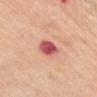Q: Was a biopsy performed?
A: imaged on a skin check; not biopsied
Q: What kind of image is this?
A: ~15 mm crop, total-body skin-cancer survey
Q: Who is the patient?
A: female, aged 63–67
Q: Where on the body is the lesion?
A: the front of the torso
Q: Lesion size?
A: ~3 mm (longest diameter)
Q: How was the tile lit?
A: white-light